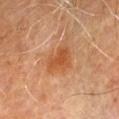Assessment:
Part of a total-body skin-imaging series; this lesion was reviewed on a skin check and was not flagged for biopsy.
Acquisition and patient details:
A male patient, about 70 years old. The lesion is located on the left upper arm. The tile uses cross-polarized illumination. A 15 mm close-up extracted from a 3D total-body photography capture. Measured at roughly 4 mm in maximum diameter.